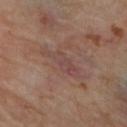Captured during whole-body skin photography for melanoma surveillance; the lesion was not biopsied. From the left lower leg. Longest diameter approximately 5.5 mm. Cropped from a total-body skin-imaging series; the visible field is about 15 mm. This is a cross-polarized tile. A female subject aged 48 to 52.